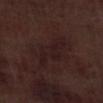Acquisition and patient details: Captured under white-light illumination. Longest diameter approximately 5.5 mm. A male patient, about 70 years old. A region of skin cropped from a whole-body photographic capture, roughly 15 mm wide. Located on the left lower leg. The total-body-photography lesion software estimated an average lesion color of about L≈18 a*≈16 b*≈14 (CIELAB) and roughly 4 lightness units darker than nearby skin. It also reported a border-irregularity index near 5/10, a within-lesion color-variation index near 2/10, and a peripheral color-asymmetry measure near 0.5. The software also gave a nevus-likeness score of about 0/100 and a lesion-detection confidence of about 80/100.